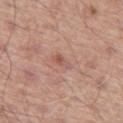* notes · total-body-photography surveillance lesion; no biopsy
* tile lighting · white-light illumination
* image source · total-body-photography crop, ~15 mm field of view
* subject · male, in their mid-60s
* site · the left thigh